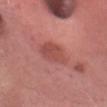| feature | finding |
|---|---|
| follow-up | total-body-photography surveillance lesion; no biopsy |
| site | the head or neck |
| patient | female, aged 23–27 |
| image | ~15 mm crop, total-body skin-cancer survey |
| lighting | white-light illumination |